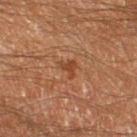The lesion was tiled from a total-body skin photograph and was not biopsied.
A male subject, roughly 60 years of age.
Longest diameter approximately 2.5 mm.
A 15 mm close-up tile from a total-body photography series done for melanoma screening.
The lesion is on the left thigh.
The total-body-photography lesion software estimated a lesion color around L≈33 a*≈20 b*≈27 in CIELAB, a lesion–skin lightness drop of about 6, and a lesion-to-skin contrast of about 6.5 (normalized; higher = more distinct). And it measured a border-irregularity rating of about 6/10 and peripheral color asymmetry of about 0.